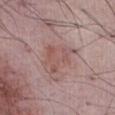| field | value |
|---|---|
| biopsy status | imaged on a skin check; not biopsied |
| patient | male, about 55 years old |
| anatomic site | the abdomen |
| image | ~15 mm crop, total-body skin-cancer survey |
| diameter | ≈5.5 mm |
| image-analysis metrics | a lesion area of about 10 mm² and a shape eccentricity near 0.75; a lesion color around L≈53 a*≈21 b*≈22 in CIELAB, roughly 7 lightness units darker than nearby skin, and a lesion-to-skin contrast of about 5.5 (normalized; higher = more distinct); a within-lesion color-variation index near 3.5/10 and a peripheral color-asymmetry measure near 1; an automated nevus-likeness rating near 0 out of 100 and lesion-presence confidence of about 100/100 |
| lighting | white-light illumination |